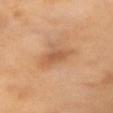{"biopsy_status": "not biopsied; imaged during a skin examination", "automated_metrics": {"lesion_detection_confidence_0_100": 100}, "lighting": "cross-polarized", "site": "chest", "image": {"source": "total-body photography crop", "field_of_view_mm": 15}, "lesion_size": {"long_diameter_mm_approx": 4.5}, "patient": {"sex": "female", "age_approx": 55}}